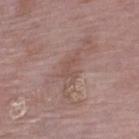notes: catalogued during a skin exam; not biopsied
lesion diameter: ≈4.5 mm
anatomic site: the left lower leg
patient: female, aged around 70
image source: 15 mm crop, total-body photography
TBP lesion metrics: a border-irregularity index near 5.5/10 and a peripheral color-asymmetry measure near 1
illumination: white-light illumination